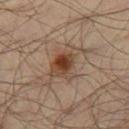Assessment: The lesion was photographed on a routine skin check and not biopsied; there is no pathology result. Background: The patient is a male in their mid-60s. This is a cross-polarized tile. This image is a 15 mm lesion crop taken from a total-body photograph. Automated tile analysis of the lesion measured a border-irregularity rating of about 7/10. The lesion is located on the right thigh. Measured at roughly 6.5 mm in maximum diameter.